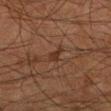biopsy status = catalogued during a skin exam; not biopsied | illumination = cross-polarized illumination | image-analysis metrics = a footprint of about 3 mm², a shape eccentricity near 0.85, and two-axis asymmetry of about 0.4; a border-irregularity index near 3.5/10, a color-variation rating of about 1/10, and peripheral color asymmetry of about 0.5; an automated nevus-likeness rating near 0 out of 100 and a detector confidence of about 80 out of 100 that the crop contains a lesion | imaging modality = ~15 mm tile from a whole-body skin photo | subject = male, aged approximately 65 | body site = the left lower leg.